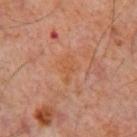– image · total-body-photography crop, ~15 mm field of view
– body site · the chest
– automated metrics · a lesion area of about 4 mm² and an outline eccentricity of about 0.85 (0 = round, 1 = elongated); a mean CIELAB color near L≈50 a*≈23 b*≈34, roughly 4 lightness units darker than nearby skin, and a normalized lesion–skin contrast near 5.5; a border-irregularity rating of about 4.5/10, a within-lesion color-variation index near 1/10, and peripheral color asymmetry of about 0.5; a nevus-likeness score of about 0/100
– patient · male, approximately 60 years of age
– diameter · ~3 mm (longest diameter)